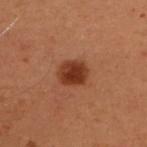<record>
<biopsy_status>not biopsied; imaged during a skin examination</biopsy_status>
<site>upper back</site>
<automated_metrics>
  <eccentricity>0.65</eccentricity>
  <shape_asymmetry>0.1</shape_asymmetry>
  <cielab_L>32</cielab_L>
  <cielab_a>23</cielab_a>
  <cielab_b>29</cielab_b>
  <vs_skin_darker_L>11.0</vs_skin_darker_L>
  <vs_skin_contrast_norm>10.0</vs_skin_contrast_norm>
</automated_metrics>
<patient>
  <sex>female</sex>
  <age_approx>50</age_approx>
</patient>
<lesion_size>
  <long_diameter_mm_approx>3.5</long_diameter_mm_approx>
</lesion_size>
<image>
  <source>total-body photography crop</source>
  <field_of_view_mm>15</field_of_view_mm>
</image>
</record>